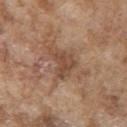Part of a total-body skin-imaging series; this lesion was reviewed on a skin check and was not flagged for biopsy. The recorded lesion diameter is about 4 mm. The total-body-photography lesion software estimated a lesion area of about 7 mm² and an outline eccentricity of about 0.65 (0 = round, 1 = elongated). And it measured a mean CIELAB color near L≈47 a*≈19 b*≈30, a lesion–skin lightness drop of about 9, and a lesion-to-skin contrast of about 6.5 (normalized; higher = more distinct). The software also gave a border-irregularity rating of about 6/10, a color-variation rating of about 2/10, and a peripheral color-asymmetry measure near 0.5. And it measured a nevus-likeness score of about 0/100 and a detector confidence of about 100 out of 100 that the crop contains a lesion. A male patient, aged approximately 75. A roughly 15 mm field-of-view crop from a total-body skin photograph. This is a white-light tile. The lesion is located on the arm.